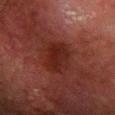Recorded during total-body skin imaging; not selected for excision or biopsy.